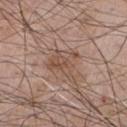Q: Is there a histopathology result?
A: imaged on a skin check; not biopsied
Q: How was this image acquired?
A: 15 mm crop, total-body photography
Q: Illumination type?
A: white-light
Q: Patient demographics?
A: male, aged approximately 65
Q: What is the lesion's diameter?
A: ~4 mm (longest diameter)
Q: Lesion location?
A: the chest